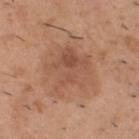Assessment:
No biopsy was performed on this lesion — it was imaged during a full skin examination and was not determined to be concerning.
Image and clinical context:
Automated image analysis of the tile measured a footprint of about 21 mm² and two-axis asymmetry of about 0.35. The software also gave a border-irregularity rating of about 5.5/10, internal color variation of about 5 on a 0–10 scale, and peripheral color asymmetry of about 2. It also reported a nevus-likeness score of about 10/100 and lesion-presence confidence of about 100/100. The recorded lesion diameter is about 6 mm. The patient is a male aged 53–57. Located on the head or neck. The tile uses white-light illumination. This image is a 15 mm lesion crop taken from a total-body photograph.